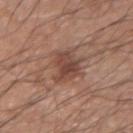{
  "image": {
    "source": "total-body photography crop",
    "field_of_view_mm": 15
  },
  "lesion_size": {
    "long_diameter_mm_approx": 4.5
  },
  "site": "left forearm",
  "lighting": "white-light",
  "patient": {
    "sex": "male",
    "age_approx": 65
  }
}